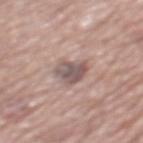<record>
  <biopsy_status>not biopsied; imaged during a skin examination</biopsy_status>
  <image>
    <source>total-body photography crop</source>
    <field_of_view_mm>15</field_of_view_mm>
  </image>
  <site>mid back</site>
  <patient>
    <sex>male</sex>
    <age_approx>75</age_approx>
  </patient>
  <lesion_size>
    <long_diameter_mm_approx>4.0</long_diameter_mm_approx>
  </lesion_size>
  <lighting>white-light</lighting>
</record>